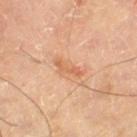Assessment: The lesion was photographed on a routine skin check and not biopsied; there is no pathology result. Context: Located on the right thigh. Longest diameter approximately 3.5 mm. The subject is a male roughly 65 years of age. A close-up tile cropped from a whole-body skin photograph, about 15 mm across. Captured under cross-polarized illumination.